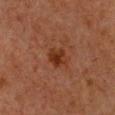| key | value |
|---|---|
| notes | imaged on a skin check; not biopsied |
| anatomic site | the chest |
| subject | female, aged 38 to 42 |
| image-analysis metrics | a border-irregularity rating of about 2.5/10, a color-variation rating of about 3/10, and a peripheral color-asymmetry measure near 1; a lesion-detection confidence of about 100/100 |
| image | ~15 mm crop, total-body skin-cancer survey |
| illumination | cross-polarized |
| size | ~3 mm (longest diameter) |